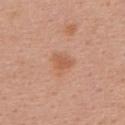Impression: The lesion was photographed on a routine skin check and not biopsied; there is no pathology result. Context: Captured under white-light illumination. The lesion is located on the upper back. Longest diameter approximately 2.5 mm. An algorithmic analysis of the crop reported roughly 8 lightness units darker than nearby skin and a normalized border contrast of about 6. And it measured border irregularity of about 3.5 on a 0–10 scale, internal color variation of about 1 on a 0–10 scale, and a peripheral color-asymmetry measure near 0.5. The patient is a female aged 38 to 42. A region of skin cropped from a whole-body photographic capture, roughly 15 mm wide.